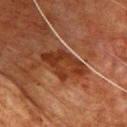notes: no biopsy performed (imaged during a skin exam) | patient: male, about 80 years old | anatomic site: the chest | TBP lesion metrics: a lesion color around L≈28 a*≈22 b*≈28 in CIELAB, roughly 9 lightness units darker than nearby skin, and a lesion-to-skin contrast of about 9 (normalized; higher = more distinct); internal color variation of about 4 on a 0–10 scale; a nevus-likeness score of about 0/100 and a detector confidence of about 85 out of 100 that the crop contains a lesion | imaging modality: total-body-photography crop, ~15 mm field of view.